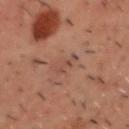workup: catalogued during a skin exam; not biopsied
automated metrics: an area of roughly 4 mm², an outline eccentricity of about 0.75 (0 = round, 1 = elongated), and two-axis asymmetry of about 0.3; a mean CIELAB color near L≈35 a*≈16 b*≈21; a border-irregularity rating of about 3.5/10, a within-lesion color-variation index near 2/10, and radial color variation of about 1; an automated nevus-likeness rating near 0 out of 100 and lesion-presence confidence of about 95/100
acquisition: total-body-photography crop, ~15 mm field of view
anatomic site: the head or neck
patient: male, in their 50s
diameter: about 3 mm
lighting: cross-polarized